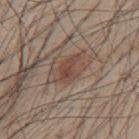Notes:
• workup: imaged on a skin check; not biopsied
• body site: the mid back
• subject: male, aged 43 to 47
• imaging modality: ~15 mm tile from a whole-body skin photo